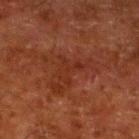notes = no biopsy performed (imaged during a skin exam)
image source = 15 mm crop, total-body photography
site = the leg
subject = male, in their 80s